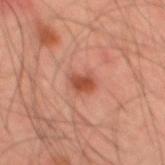Clinical impression:
Imaged during a routine full-body skin examination; the lesion was not biopsied and no histopathology is available.
Clinical summary:
Located on the mid back. A male subject aged 43–47. Cropped from a total-body skin-imaging series; the visible field is about 15 mm.